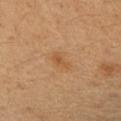* automated metrics: a lesion area of about 3 mm²; a classifier nevus-likeness of about 10/100 and lesion-presence confidence of about 100/100
* imaging modality: 15 mm crop, total-body photography
* subject: female, in their 50s
* lesion diameter: ≈2.5 mm
* site: the left forearm